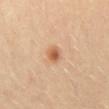Recorded during total-body skin imaging; not selected for excision or biopsy. The total-body-photography lesion software estimated a symmetry-axis asymmetry near 0.15. And it measured a mean CIELAB color near L≈56 a*≈22 b*≈35, about 11 CIELAB-L* units darker than the surrounding skin, and a lesion-to-skin contrast of about 8 (normalized; higher = more distinct). It also reported a border-irregularity rating of about 1.5/10, internal color variation of about 4 on a 0–10 scale, and a peripheral color-asymmetry measure near 1.5. The analysis additionally found a nevus-likeness score of about 95/100. The subject is a male in their 20s. The lesion is on the abdomen. A lesion tile, about 15 mm wide, cut from a 3D total-body photograph.